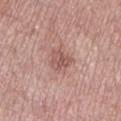| feature | finding |
|---|---|
| workup | catalogued during a skin exam; not biopsied |
| lesion diameter | about 2.5 mm |
| subject | female, approximately 70 years of age |
| location | the left lower leg |
| image source | ~15 mm crop, total-body skin-cancer survey |